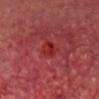Image and clinical context: On the head or neck. A male subject aged approximately 60. A close-up tile cropped from a whole-body skin photograph, about 15 mm across. Pathology: The biopsy diagnosis was an invasive squamous cell carcinoma (malignant).